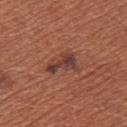  biopsy_status: not biopsied; imaged during a skin examination
  lesion_size:
    long_diameter_mm_approx: 4.0
  patient:
    sex: female
    age_approx: 35
  lighting: white-light
  site: arm
  image:
    source: total-body photography crop
    field_of_view_mm: 15